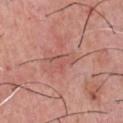Q: Lesion size?
A: ≈3.5 mm
Q: What did automated image analysis measure?
A: an eccentricity of roughly 0.8 and a symmetry-axis asymmetry near 0.35; a mean CIELAB color near L≈55 a*≈26 b*≈26, roughly 6 lightness units darker than nearby skin, and a lesion-to-skin contrast of about 4.5 (normalized; higher = more distinct)
Q: Patient demographics?
A: male, aged 53 to 57
Q: How was this image acquired?
A: ~15 mm crop, total-body skin-cancer survey
Q: Where on the body is the lesion?
A: the front of the torso
Q: Illumination type?
A: white-light